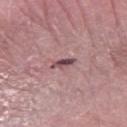biopsy status = total-body-photography surveillance lesion; no biopsy | patient = male, aged around 70 | lesion diameter = ≈2.5 mm | image = 15 mm crop, total-body photography | tile lighting = white-light illumination | site = the left forearm | TBP lesion metrics = a shape eccentricity near 0.9 and two-axis asymmetry of about 0.3; a border-irregularity index near 3/10, internal color variation of about 4 on a 0–10 scale, and peripheral color asymmetry of about 1.5; a classifier nevus-likeness of about 10/100 and a detector confidence of about 75 out of 100 that the crop contains a lesion.